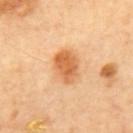Q: What is the anatomic site?
A: the mid back
Q: What kind of image is this?
A: 15 mm crop, total-body photography
Q: Illumination type?
A: cross-polarized